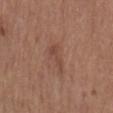<record>
<biopsy_status>not biopsied; imaged during a skin examination</biopsy_status>
<automated_metrics>
  <cielab_L>46</cielab_L>
  <cielab_a>21</cielab_a>
  <cielab_b>27</cielab_b>
  <vs_skin_contrast_norm>5.0</vs_skin_contrast_norm>
  <border_irregularity_0_10>5.0</border_irregularity_0_10>
  <color_variation_0_10>1.0</color_variation_0_10>
  <peripheral_color_asymmetry>0.5</peripheral_color_asymmetry>
  <nevus_likeness_0_100>0</nevus_likeness_0_100>
</automated_metrics>
<lighting>white-light</lighting>
<image>
  <source>total-body photography crop</source>
  <field_of_view_mm>15</field_of_view_mm>
</image>
<lesion_size>
  <long_diameter_mm_approx>4.0</long_diameter_mm_approx>
</lesion_size>
<site>mid back</site>
<patient>
  <sex>female</sex>
  <age_approx>65</age_approx>
</patient>
</record>